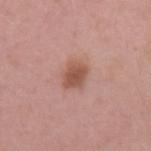Impression:
Part of a total-body skin-imaging series; this lesion was reviewed on a skin check and was not flagged for biopsy.
Image and clinical context:
On the left upper arm. Automated image analysis of the tile measured an average lesion color of about L≈53 a*≈23 b*≈27 (CIELAB), about 11 CIELAB-L* units darker than the surrounding skin, and a normalized lesion–skin contrast near 8. The software also gave a classifier nevus-likeness of about 85/100 and a detector confidence of about 100 out of 100 that the crop contains a lesion. Approximately 3 mm at its widest. The tile uses white-light illumination. A 15 mm close-up tile from a total-body photography series done for melanoma screening. The patient is a female about 45 years old.